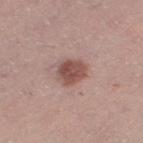Notes:
• biopsy status · total-body-photography surveillance lesion; no biopsy
• imaging modality · 15 mm crop, total-body photography
• subject · female, aged 38–42
• site · the left thigh
• tile lighting · white-light illumination
• lesion size · ≈3.5 mm